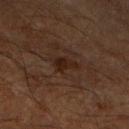Part of a total-body skin-imaging series; this lesion was reviewed on a skin check and was not flagged for biopsy. On the arm. A male subject aged around 65. Measured at roughly 2.5 mm in maximum diameter. A lesion tile, about 15 mm wide, cut from a 3D total-body photograph. Captured under cross-polarized illumination.